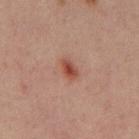  biopsy_status: not biopsied; imaged during a skin examination